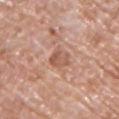Notes:
– follow-up: no biopsy performed (imaged during a skin exam)
– automated metrics: a classifier nevus-likeness of about 0/100 and a lesion-detection confidence of about 100/100
– location: the right upper arm
– patient: male, roughly 70 years of age
– acquisition: ~15 mm tile from a whole-body skin photo